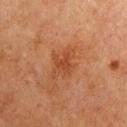- workup: catalogued during a skin exam; not biopsied
- image source: 15 mm crop, total-body photography
- subject: female, about 50 years old
- size: about 4 mm
- location: the chest
- automated metrics: an area of roughly 7.5 mm² and two-axis asymmetry of about 0.3; a border-irregularity index near 4/10, a color-variation rating of about 2.5/10, and radial color variation of about 0.5; an automated nevus-likeness rating near 5 out of 100 and a detector confidence of about 100 out of 100 that the crop contains a lesion